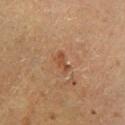| field | value |
|---|---|
| follow-up | total-body-photography surveillance lesion; no biopsy |
| anatomic site | the leg |
| image source | 15 mm crop, total-body photography |
| subject | female, roughly 50 years of age |
| illumination | cross-polarized illumination |